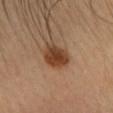Q: Is there a histopathology result?
A: total-body-photography surveillance lesion; no biopsy
Q: Lesion location?
A: the head or neck
Q: Patient demographics?
A: female, roughly 35 years of age
Q: How was this image acquired?
A: total-body-photography crop, ~15 mm field of view